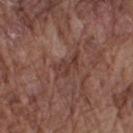Findings:
* notes · total-body-photography surveillance lesion; no biopsy
* subject · male, aged approximately 75
* acquisition · ~15 mm crop, total-body skin-cancer survey
* tile lighting · white-light illumination
* body site · the right upper arm
* TBP lesion metrics · a border-irregularity index near 5/10, a within-lesion color-variation index near 2/10, and a peripheral color-asymmetry measure near 0.5
* size · ~3.5 mm (longest diameter)